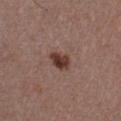Recorded during total-body skin imaging; not selected for excision or biopsy. A region of skin cropped from a whole-body photographic capture, roughly 15 mm wide. A male subject, aged 38–42. On the chest. Imaged with white-light lighting.